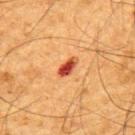  biopsy_status: not biopsied; imaged during a skin examination
  site: upper back
  patient:
    sex: male
    age_approx: 65
  image:
    source: total-body photography crop
    field_of_view_mm: 15